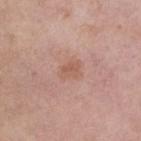The lesion was tiled from a total-body skin photograph and was not biopsied. The lesion is on the right lower leg. The lesion's longest dimension is about 2.5 mm. A female patient aged 48 to 52. A lesion tile, about 15 mm wide, cut from a 3D total-body photograph. Imaged with white-light lighting. The lesion-visualizer software estimated an average lesion color of about L≈57 a*≈22 b*≈28 (CIELAB), a lesion–skin lightness drop of about 7, and a normalized lesion–skin contrast near 5.5.